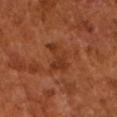Impression: Captured during whole-body skin photography for melanoma surveillance; the lesion was not biopsied. Context: A male patient, approximately 65 years of age. A 15 mm close-up extracted from a 3D total-body photography capture.